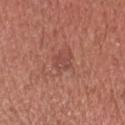Notes:
– anatomic site · the head or neck
– acquisition · 15 mm crop, total-body photography
– patient · male, aged approximately 45